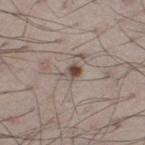Captured during whole-body skin photography for melanoma surveillance; the lesion was not biopsied. About 2.5 mm across. The tile uses white-light illumination. A 15 mm crop from a total-body photograph taken for skin-cancer surveillance. A male patient, aged around 65. From the left thigh.